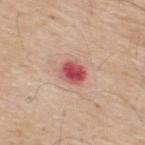notes: imaged on a skin check; not biopsied
site: the upper back
image: 15 mm crop, total-body photography
subject: male, approximately 70 years of age